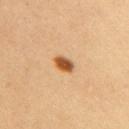Q: Is there a histopathology result?
A: total-body-photography surveillance lesion; no biopsy
Q: What are the patient's age and sex?
A: female, about 30 years old
Q: Lesion size?
A: about 2.5 mm
Q: What is the imaging modality?
A: 15 mm crop, total-body photography
Q: What is the anatomic site?
A: the right upper arm